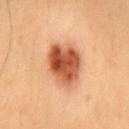Clinical impression: Captured during whole-body skin photography for melanoma surveillance; the lesion was not biopsied. Acquisition and patient details: The tile uses cross-polarized illumination. Measured at roughly 5 mm in maximum diameter. From the back. Cropped from a total-body skin-imaging series; the visible field is about 15 mm. A male subject, in their mid-50s.